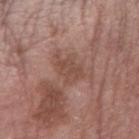site: right forearm
image:
  source: total-body photography crop
  field_of_view_mm: 15
patient:
  sex: male
  age_approx: 75
automated_metrics:
  area_mm2_approx: 7.0
  eccentricity: 0.65
  shape_asymmetry: 0.35
  border_irregularity_0_10: 4.5
  peripheral_color_asymmetry: 1.0
lesion_size:
  long_diameter_mm_approx: 3.5